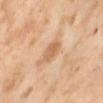biopsy_status: not biopsied; imaged during a skin examination
lesion_size:
  long_diameter_mm_approx: 2.5
site: front of the torso
lighting: cross-polarized
image:
  source: total-body photography crop
  field_of_view_mm: 15
patient:
  sex: female
  age_approx: 55